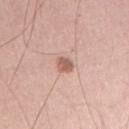<record>
  <biopsy_status>not biopsied; imaged during a skin examination</biopsy_status>
  <lighting>white-light</lighting>
  <lesion_size>
    <long_diameter_mm_approx>2.5</long_diameter_mm_approx>
  </lesion_size>
  <image>
    <source>total-body photography crop</source>
    <field_of_view_mm>15</field_of_view_mm>
  </image>
  <site>right upper arm</site>
  <patient>
    <sex>male</sex>
    <age_approx>40</age_approx>
  </patient>
</record>